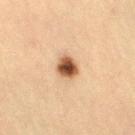Captured during whole-body skin photography for melanoma surveillance; the lesion was not biopsied.
About 2.5 mm across.
The subject is a male aged 58 to 62.
Located on the right thigh.
A 15 mm close-up tile from a total-body photography series done for melanoma screening.